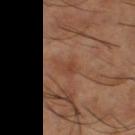Imaged during a routine full-body skin examination; the lesion was not biopsied and no histopathology is available.
This image is a 15 mm lesion crop taken from a total-body photograph.
Automated tile analysis of the lesion measured a footprint of about 4.5 mm², an eccentricity of roughly 0.85, and a shape-asymmetry score of about 0.25 (0 = symmetric). The analysis additionally found an automated nevus-likeness rating near 0 out of 100 and a detector confidence of about 100 out of 100 that the crop contains a lesion.
The lesion is located on the leg.
Longest diameter approximately 3 mm.
A male patient, aged around 65.
Imaged with cross-polarized lighting.